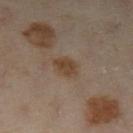follow-up: no biopsy performed (imaged during a skin exam) | automated metrics: border irregularity of about 2 on a 0–10 scale, a within-lesion color-variation index near 2/10, and a peripheral color-asymmetry measure near 1; an automated nevus-likeness rating near 65 out of 100 and lesion-presence confidence of about 100/100 | location: the right leg | subject: female, aged around 60 | acquisition: total-body-photography crop, ~15 mm field of view.